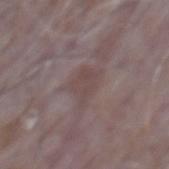Clinical impression:
Captured during whole-body skin photography for melanoma surveillance; the lesion was not biopsied.
Context:
Automated tile analysis of the lesion measured a lesion color around L≈44 a*≈15 b*≈17 in CIELAB, about 6 CIELAB-L* units darker than the surrounding skin, and a normalized lesion–skin contrast near 5.5. The analysis additionally found internal color variation of about 1.5 on a 0–10 scale and peripheral color asymmetry of about 0.5. This is a white-light tile. On the mid back. Measured at roughly 3 mm in maximum diameter. A 15 mm close-up extracted from a 3D total-body photography capture. The patient is a male aged approximately 65.